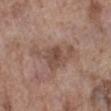The lesion was tiled from a total-body skin photograph and was not biopsied.
The lesion is located on the left lower leg.
Cropped from a total-body skin-imaging series; the visible field is about 15 mm.
A female patient in their mid-80s or older.
Approximately 6.5 mm at its widest.
Captured under white-light illumination.